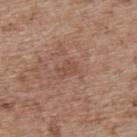<tbp_lesion>
  <biopsy_status>not biopsied; imaged during a skin examination</biopsy_status>
  <lesion_size>
    <long_diameter_mm_approx>2.5</long_diameter_mm_approx>
  </lesion_size>
  <site>upper back</site>
  <image>
    <source>total-body photography crop</source>
    <field_of_view_mm>15</field_of_view_mm>
  </image>
  <lighting>white-light</lighting>
  <patient>
    <sex>male</sex>
    <age_approx>70</age_approx>
  </patient>
</tbp_lesion>